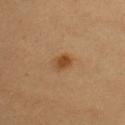Notes:
- follow-up: total-body-photography surveillance lesion; no biopsy
- acquisition: ~15 mm tile from a whole-body skin photo
- lesion size: ~2 mm (longest diameter)
- location: the chest
- patient: female, aged 38–42